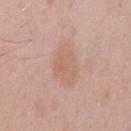Part of a total-body skin-imaging series; this lesion was reviewed on a skin check and was not flagged for biopsy. The lesion is located on the chest. Cropped from a total-body skin-imaging series; the visible field is about 15 mm. Imaged with white-light lighting. The subject is a male aged 48–52. The recorded lesion diameter is about 5.5 mm.